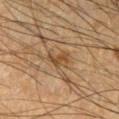Clinical impression: Recorded during total-body skin imaging; not selected for excision or biopsy. Image and clinical context: A roughly 15 mm field-of-view crop from a total-body skin photograph. A male subject aged approximately 60. The lesion is located on the left forearm.